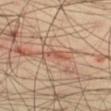Recorded during total-body skin imaging; not selected for excision or biopsy. A lesion tile, about 15 mm wide, cut from a 3D total-body photograph. A male subject aged around 40. Automated tile analysis of the lesion measured an area of roughly 3.5 mm², an eccentricity of roughly 0.9, and a shape-asymmetry score of about 0.45 (0 = symmetric). And it measured an average lesion color of about L≈55 a*≈24 b*≈30 (CIELAB), roughly 9 lightness units darker than nearby skin, and a normalized lesion–skin contrast near 6. And it measured a border-irregularity rating of about 4.5/10, a color-variation rating of about 1/10, and peripheral color asymmetry of about 0.5. The analysis additionally found a lesion-detection confidence of about 85/100. From the right thigh. About 3 mm across.